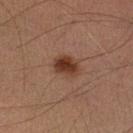The lesion was tiled from a total-body skin photograph and was not biopsied.
This image is a 15 mm lesion crop taken from a total-body photograph.
The lesion is on the right leg.
Captured under cross-polarized illumination.
A male subject aged approximately 35.
Approximately 3 mm at its widest.
Automated image analysis of the tile measured two-axis asymmetry of about 0.2. And it measured a mean CIELAB color near L≈35 a*≈21 b*≈28, a lesion–skin lightness drop of about 12, and a normalized lesion–skin contrast near 10.5. The analysis additionally found a border-irregularity index near 1.5/10 and internal color variation of about 3.5 on a 0–10 scale.